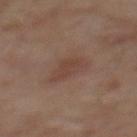Findings:
- follow-up: no biopsy performed (imaged during a skin exam)
- illumination: cross-polarized
- patient: male, aged 63–67
- location: the back
- size: ≈4.5 mm
- image: ~15 mm tile from a whole-body skin photo